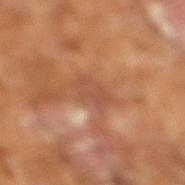  biopsy_status: not biopsied; imaged during a skin examination
  automated_metrics:
    eccentricity: 0.75
    border_irregularity_0_10: 5.0
    color_variation_0_10: 2.0
    peripheral_color_asymmetry: 0.5
  patient:
    sex: male
    age_approx: 65
  image:
    source: total-body photography crop
    field_of_view_mm: 15
  lighting: cross-polarized
  lesion_size:
    long_diameter_mm_approx: 3.0